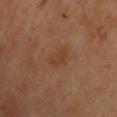The lesion was photographed on a routine skin check and not biopsied; there is no pathology result. A roughly 15 mm field-of-view crop from a total-body skin photograph. The patient is a male aged around 70. On the upper back. Longest diameter approximately 4 mm. Imaged with cross-polarized lighting.